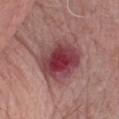Assessment: Captured during whole-body skin photography for melanoma surveillance; the lesion was not biopsied. Image and clinical context: A male patient, roughly 65 years of age. From the chest. A 15 mm close-up tile from a total-body photography series done for melanoma screening. Automated tile analysis of the lesion measured a lesion area of about 26 mm², a shape eccentricity near 0.5, and two-axis asymmetry of about 0.25. The software also gave an average lesion color of about L≈43 a*≈27 b*≈19 (CIELAB) and a normalized border contrast of about 10.5. And it measured an automated nevus-likeness rating near 0 out of 100 and a detector confidence of about 100 out of 100 that the crop contains a lesion.